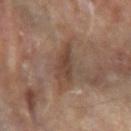Impression:
No biopsy was performed on this lesion — it was imaged during a full skin examination and was not determined to be concerning.
Clinical summary:
Automated tile analysis of the lesion measured an area of roughly 8.5 mm² and an eccentricity of roughly 0.85. It also reported an average lesion color of about L≈41 a*≈16 b*≈25 (CIELAB), a lesion–skin lightness drop of about 8, and a normalized lesion–skin contrast near 7. The analysis additionally found an automated nevus-likeness rating near 15 out of 100 and a detector confidence of about 95 out of 100 that the crop contains a lesion. A 15 mm close-up tile from a total-body photography series done for melanoma screening. The lesion is located on the left forearm. About 4.5 mm across. A male subject, in their mid- to late 80s or older. This is a cross-polarized tile.